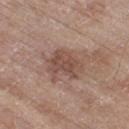This lesion was catalogued during total-body skin photography and was not selected for biopsy.
The subject is a male about 80 years old.
The recorded lesion diameter is about 4.5 mm.
A lesion tile, about 15 mm wide, cut from a 3D total-body photograph.
The lesion is on the right thigh.
The tile uses white-light illumination.